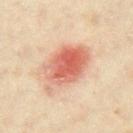No biopsy was performed on this lesion — it was imaged during a full skin examination and was not determined to be concerning. An algorithmic analysis of the crop reported a footprint of about 21 mm² and an eccentricity of roughly 0.7. It also reported a border-irregularity index near 2.5/10, a within-lesion color-variation index near 8/10, and radial color variation of about 2.5. The analysis additionally found a nevus-likeness score of about 95/100. On the leg. The tile uses cross-polarized illumination. A lesion tile, about 15 mm wide, cut from a 3D total-body photograph. A female patient aged around 35. Longest diameter approximately 7 mm.